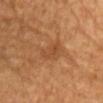biopsy status: total-body-photography surveillance lesion; no biopsy | patient: male, approximately 85 years of age or older | image source: ~15 mm tile from a whole-body skin photo | automated lesion analysis: a border-irregularity rating of about 2.5/10, a within-lesion color-variation index near 3/10, and radial color variation of about 1; a detector confidence of about 100 out of 100 that the crop contains a lesion | anatomic site: the chest.